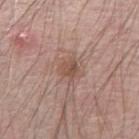The lesion was photographed on a routine skin check and not biopsied; there is no pathology result. About 2.5 mm across. The lesion-visualizer software estimated a lesion area of about 4 mm² and two-axis asymmetry of about 0.3. It also reported peripheral color asymmetry of about 1.5. Cropped from a whole-body photographic skin survey; the tile spans about 15 mm. From the right upper arm. Captured under white-light illumination. A male patient, roughly 70 years of age.